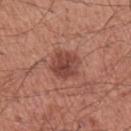A male patient aged 58–62.
A 15 mm close-up tile from a total-body photography series done for melanoma screening.
The lesion is located on the right upper arm.